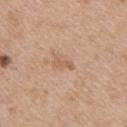<tbp_lesion>
<biopsy_status>not biopsied; imaged during a skin examination</biopsy_status>
<image>
  <source>total-body photography crop</source>
  <field_of_view_mm>15</field_of_view_mm>
</image>
<site>upper back</site>
<lighting>white-light</lighting>
<patient>
  <sex>female</sex>
  <age_approx>45</age_approx>
</patient>
</tbp_lesion>